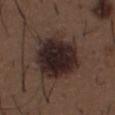{"biopsy_status": "not biopsied; imaged during a skin examination", "lesion_size": {"long_diameter_mm_approx": 6.5}, "image": {"source": "total-body photography crop", "field_of_view_mm": 15}, "site": "abdomen", "lighting": "white-light", "patient": {"sex": "male", "age_approx": 50}, "automated_metrics": {"area_mm2_approx": 27.0, "eccentricity": 0.65, "shape_asymmetry": 0.2, "cielab_L": 24, "cielab_a": 13, "cielab_b": 15, "vs_skin_contrast_norm": 15.0, "nevus_likeness_0_100": 85, "lesion_detection_confidence_0_100": 100}}